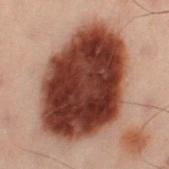Clinical impression:
Recorded during total-body skin imaging; not selected for excision or biopsy.
Context:
A male subject aged 48 to 52. Captured under cross-polarized illumination. On the left thigh. The lesion's longest dimension is about 11 mm. Cropped from a total-body skin-imaging series; the visible field is about 15 mm.